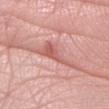notes: catalogued during a skin exam; not biopsied
illumination: white-light
patient: male, aged approximately 40
anatomic site: the right forearm
image source: 15 mm crop, total-body photography